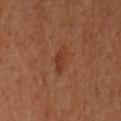The lesion was tiled from a total-body skin photograph and was not biopsied. Imaged with cross-polarized lighting. A lesion tile, about 15 mm wide, cut from a 3D total-body photograph. Longest diameter approximately 3 mm. The lesion is on the right arm. Automated tile analysis of the lesion measured a footprint of about 4.5 mm², an eccentricity of roughly 0.75, and two-axis asymmetry of about 0.2. The analysis additionally found roughly 6 lightness units darker than nearby skin and a lesion-to-skin contrast of about 5.5 (normalized; higher = more distinct). A female patient aged 58–62.